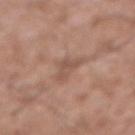Captured during whole-body skin photography for melanoma surveillance; the lesion was not biopsied. This is a white-light tile. From the abdomen. Cropped from a whole-body photographic skin survey; the tile spans about 15 mm. A male patient aged approximately 75.